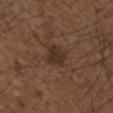This lesion was catalogued during total-body skin photography and was not selected for biopsy. Cropped from a whole-body photographic skin survey; the tile spans about 15 mm. This is a white-light tile. Longest diameter approximately 3 mm. On the left upper arm. The patient is a male aged 48 to 52.